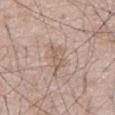{
  "biopsy_status": "not biopsied; imaged during a skin examination",
  "automated_metrics": {
    "area_mm2_approx": 5.0,
    "shape_asymmetry": 0.35,
    "cielab_L": 59,
    "cielab_a": 16,
    "cielab_b": 26,
    "vs_skin_contrast_norm": 5.5,
    "nevus_likeness_0_100": 0,
    "lesion_detection_confidence_0_100": 70
  },
  "lesion_size": {
    "long_diameter_mm_approx": 3.5
  },
  "site": "abdomen",
  "image": {
    "source": "total-body photography crop",
    "field_of_view_mm": 15
  },
  "patient": {
    "sex": "male",
    "age_approx": 50
  }
}